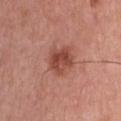Case summary:
• workup: imaged on a skin check; not biopsied
• TBP lesion metrics: an area of roughly 8 mm², an outline eccentricity of about 0.55 (0 = round, 1 = elongated), and a shape-asymmetry score of about 0.2 (0 = symmetric); a border-irregularity rating of about 2/10 and a peripheral color-asymmetry measure near 1.5
• subject: male, aged 58–62
• acquisition: 15 mm crop, total-body photography
• size: ≈3.5 mm
• site: the chest
• illumination: white-light